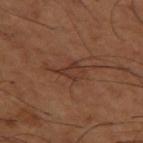| feature | finding |
|---|---|
| follow-up | total-body-photography surveillance lesion; no biopsy |
| location | the left thigh |
| subject | male, approximately 65 years of age |
| acquisition | 15 mm crop, total-body photography |